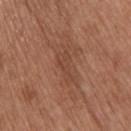notes — catalogued during a skin exam; not biopsied | lighting — white-light illumination | automated lesion analysis — a border-irregularity index near 5.5/10 and a within-lesion color-variation index near 1/10; an automated nevus-likeness rating near 0 out of 100 | patient — male, roughly 65 years of age | acquisition — 15 mm crop, total-body photography | site — the upper back.